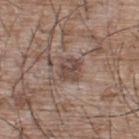Q: Was a biopsy performed?
A: catalogued during a skin exam; not biopsied
Q: What kind of image is this?
A: 15 mm crop, total-body photography
Q: Lesion size?
A: about 3 mm
Q: Lesion location?
A: the back
Q: What did automated image analysis measure?
A: a mean CIELAB color near L≈46 a*≈16 b*≈22, roughly 10 lightness units darker than nearby skin, and a normalized border contrast of about 7.5; lesion-presence confidence of about 95/100
Q: What lighting was used for the tile?
A: white-light
Q: Who is the patient?
A: male, aged around 65